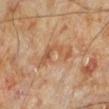biopsy_status: not biopsied; imaged during a skin examination
lighting: cross-polarized
lesion_size:
  long_diameter_mm_approx: 5.5
site: left lower leg
automated_metrics:
  area_mm2_approx: 9.0
  eccentricity: 0.9
  shape_asymmetry: 0.65
  nevus_likeness_0_100: 0
patient:
  sex: male
  age_approx: 70
image:
  source: total-body photography crop
  field_of_view_mm: 15